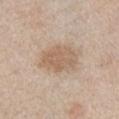This lesion was catalogued during total-body skin photography and was not selected for biopsy.